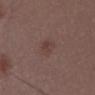The lesion was photographed on a routine skin check and not biopsied; there is no pathology result. The total-body-photography lesion software estimated a mean CIELAB color near L≈39 a*≈17 b*≈20 and about 5 CIELAB-L* units darker than the surrounding skin. The analysis additionally found a border-irregularity rating of about 3/10, a within-lesion color-variation index near 2/10, and a peripheral color-asymmetry measure near 1. And it measured an automated nevus-likeness rating near 5 out of 100 and a lesion-detection confidence of about 100/100. The patient is a male aged 48–52. This image is a 15 mm lesion crop taken from a total-body photograph. Located on the lower back. The lesion's longest dimension is about 3 mm.